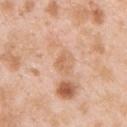Q: Is there a histopathology result?
A: total-body-photography surveillance lesion; no biopsy
Q: Patient demographics?
A: male, aged 23–27
Q: What kind of image is this?
A: 15 mm crop, total-body photography
Q: How was the tile lit?
A: white-light illumination
Q: What is the anatomic site?
A: the upper back
Q: Automated lesion metrics?
A: an average lesion color of about L≈65 a*≈21 b*≈35 (CIELAB); a color-variation rating of about 1.5/10 and a peripheral color-asymmetry measure near 0.5